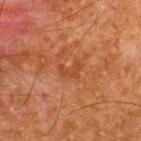Assessment: No biopsy was performed on this lesion — it was imaged during a full skin examination and was not determined to be concerning. Context: Cropped from a total-body skin-imaging series; the visible field is about 15 mm. The recorded lesion diameter is about 2.5 mm. The patient is a male aged 63–67. Located on the upper back. Imaged with cross-polarized lighting. Automated tile analysis of the lesion measured a footprint of about 3.5 mm², a shape eccentricity near 0.8, and two-axis asymmetry of about 0.5. The software also gave a lesion color around L≈37 a*≈24 b*≈33 in CIELAB, a lesion–skin lightness drop of about 5, and a normalized border contrast of about 5. The analysis additionally found border irregularity of about 5.5 on a 0–10 scale, internal color variation of about 0 on a 0–10 scale, and peripheral color asymmetry of about 0.